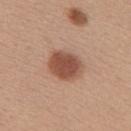| feature | finding |
|---|---|
| size | ~4 mm (longest diameter) |
| acquisition | ~15 mm tile from a whole-body skin photo |
| subject | female, aged approximately 40 |
| tile lighting | white-light illumination |
| location | the upper back |